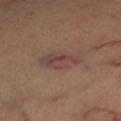biopsy status = catalogued during a skin exam; not biopsied | acquisition = ~15 mm crop, total-body skin-cancer survey | lesion diameter = ~5.5 mm (longest diameter) | anatomic site = the left lower leg | patient = female, aged 58–62.